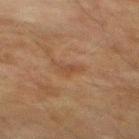Case summary:
• lesion size — about 2.5 mm
• imaging modality — 15 mm crop, total-body photography
• image-analysis metrics — roughly 5 lightness units darker than nearby skin and a normalized lesion–skin contrast near 5
• patient — male, aged 73 to 77
• location — the mid back